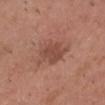Captured during whole-body skin photography for melanoma surveillance; the lesion was not biopsied.
A 15 mm close-up tile from a total-body photography series done for melanoma screening.
A male subject, aged 53 to 57.
The tile uses white-light illumination.
Located on the head or neck.
Measured at roughly 4.5 mm in maximum diameter.